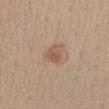Notes:
- workup: imaged on a skin check; not biopsied
- image: ~15 mm crop, total-body skin-cancer survey
- anatomic site: the front of the torso
- patient: female, approximately 45 years of age
- tile lighting: white-light